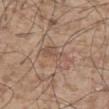biopsy status = catalogued during a skin exam; not biopsied
lesion diameter = ≈4.5 mm
image source = 15 mm crop, total-body photography
location = the abdomen
illumination = white-light
patient = male, aged 53–57